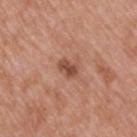Assessment:
Part of a total-body skin-imaging series; this lesion was reviewed on a skin check and was not flagged for biopsy.
Context:
Located on the back. About 2.5 mm across. The subject is a male roughly 50 years of age. This is a white-light tile. Cropped from a total-body skin-imaging series; the visible field is about 15 mm.